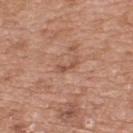Notes:
- notes — imaged on a skin check; not biopsied